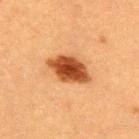follow-up = catalogued during a skin exam; not biopsied | size = about 5 mm | subject = male, in their 50s | TBP lesion metrics = a border-irregularity index near 2/10, a within-lesion color-variation index near 4.5/10, and peripheral color asymmetry of about 1; an automated nevus-likeness rating near 100 out of 100 and a detector confidence of about 100 out of 100 that the crop contains a lesion | image source = total-body-photography crop, ~15 mm field of view | location = the upper back.